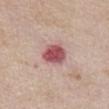Impression: Part of a total-body skin-imaging series; this lesion was reviewed on a skin check and was not flagged for biopsy. Context: The lesion is located on the chest. A female patient, approximately 75 years of age. Imaged with white-light lighting. Approximately 3.5 mm at its widest. A 15 mm crop from a total-body photograph taken for skin-cancer surveillance. An algorithmic analysis of the crop reported a nevus-likeness score of about 0/100.